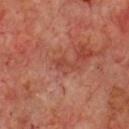• biopsy status · no biopsy performed (imaged during a skin exam)